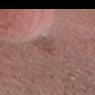Q: Was this lesion biopsied?
A: catalogued during a skin exam; not biopsied
Q: Patient demographics?
A: male, in their mid- to late 30s
Q: Where on the body is the lesion?
A: the head or neck
Q: Lesion size?
A: ≈3 mm
Q: What is the imaging modality?
A: total-body-photography crop, ~15 mm field of view